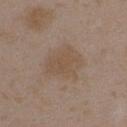{
  "biopsy_status": "not biopsied; imaged during a skin examination",
  "image": {
    "source": "total-body photography crop",
    "field_of_view_mm": 15
  },
  "lighting": "white-light",
  "lesion_size": {
    "long_diameter_mm_approx": 4.5
  },
  "patient": {
    "sex": "female",
    "age_approx": 35
  },
  "site": "right upper arm"
}